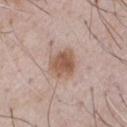<record>
  <lesion_size>
    <long_diameter_mm_approx>3.5</long_diameter_mm_approx>
  </lesion_size>
  <patient>
    <sex>male</sex>
    <age_approx>55</age_approx>
  </patient>
  <lighting>white-light</lighting>
  <automated_metrics>
    <nevus_likeness_0_100>95</nevus_likeness_0_100>
    <lesion_detection_confidence_0_100>100</lesion_detection_confidence_0_100>
  </automated_metrics>
  <image>
    <source>total-body photography crop</source>
    <field_of_view_mm>15</field_of_view_mm>
  </image>
</record>